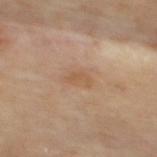Q: Is there a histopathology result?
A: total-body-photography surveillance lesion; no biopsy
Q: Where on the body is the lesion?
A: the mid back
Q: How was this image acquired?
A: ~15 mm tile from a whole-body skin photo